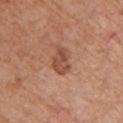biopsy status: no biopsy performed (imaged during a skin exam); lesion size: ~3.5 mm (longest diameter); illumination: white-light; image source: 15 mm crop, total-body photography; subject: female, aged around 75; site: the front of the torso.